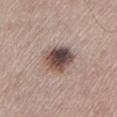follow-up: catalogued during a skin exam; not biopsied | location: the leg | lesion diameter: ≈4 mm | lighting: white-light | image: ~15 mm crop, total-body skin-cancer survey | subject: male, about 60 years old.